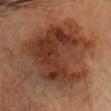Background: The lesion is located on the head or neck. The recorded lesion diameter is about 11.5 mm. A region of skin cropped from a whole-body photographic capture, roughly 15 mm wide. A male patient, roughly 55 years of age. The tile uses cross-polarized illumination.